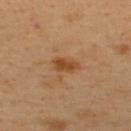Clinical impression: The lesion was photographed on a routine skin check and not biopsied; there is no pathology result.